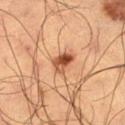The recorded lesion diameter is about 3 mm.
Located on the right thigh.
The lesion-visualizer software estimated an area of roughly 5 mm², an outline eccentricity of about 0.8 (0 = round, 1 = elongated), and a shape-asymmetry score of about 0.3 (0 = symmetric). It also reported a lesion color around L≈41 a*≈22 b*≈30 in CIELAB, roughly 13 lightness units darker than nearby skin, and a normalized lesion–skin contrast near 10.5. The analysis additionally found a border-irregularity index near 3/10, a color-variation rating of about 8.5/10, and peripheral color asymmetry of about 3.5. The analysis additionally found a classifier nevus-likeness of about 95/100 and a lesion-detection confidence of about 100/100.
The patient is a male approximately 65 years of age.
A close-up tile cropped from a whole-body skin photograph, about 15 mm across.